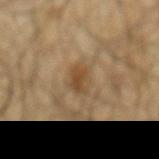Q: Was a biopsy performed?
A: catalogued during a skin exam; not biopsied
Q: Lesion size?
A: ≈3.5 mm
Q: What lighting was used for the tile?
A: cross-polarized
Q: Patient demographics?
A: male, in their mid- to late 60s
Q: What is the imaging modality?
A: ~15 mm crop, total-body skin-cancer survey
Q: Lesion location?
A: the mid back